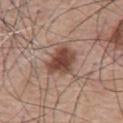Impression:
This lesion was catalogued during total-body skin photography and was not selected for biopsy.
Clinical summary:
The subject is a male aged approximately 75. Longest diameter approximately 4 mm. A roughly 15 mm field-of-view crop from a total-body skin photograph. Automated tile analysis of the lesion measured a symmetry-axis asymmetry near 0.3. It also reported an average lesion color of about L≈45 a*≈21 b*≈26 (CIELAB), a lesion–skin lightness drop of about 14, and a lesion-to-skin contrast of about 10.5 (normalized; higher = more distinct). It also reported an automated nevus-likeness rating near 95 out of 100. On the chest.